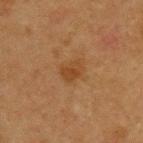Part of a total-body skin-imaging series; this lesion was reviewed on a skin check and was not flagged for biopsy.
A male patient, roughly 75 years of age.
On the upper back.
This image is a 15 mm lesion crop taken from a total-body photograph.